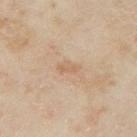notes=total-body-photography surveillance lesion; no biopsy
patient=female, approximately 35 years of age
lesion size=~2.5 mm (longest diameter)
site=the left upper arm
acquisition=total-body-photography crop, ~15 mm field of view